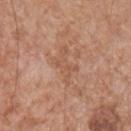The lesion was tiled from a total-body skin photograph and was not biopsied. A roughly 15 mm field-of-view crop from a total-body skin photograph. The lesion is located on the chest. Measured at roughly 4.5 mm in maximum diameter. Automated tile analysis of the lesion measured an area of roughly 7 mm², an outline eccentricity of about 0.85 (0 = round, 1 = elongated), and two-axis asymmetry of about 0.5. The analysis additionally found about 6 CIELAB-L* units darker than the surrounding skin and a lesion-to-skin contrast of about 4.5 (normalized; higher = more distinct). The analysis additionally found a nevus-likeness score of about 0/100 and lesion-presence confidence of about 95/100. A male patient aged 63–67. Captured under white-light illumination.